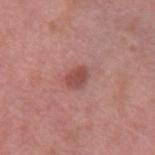Notes:
• follow-up — imaged on a skin check; not biopsied
• subject — female, aged around 40
• illumination — white-light illumination
• diameter — about 2.5 mm
• image-analysis metrics — a lesion color around L≈48 a*≈26 b*≈24 in CIELAB, roughly 10 lightness units darker than nearby skin, and a lesion-to-skin contrast of about 7.5 (normalized; higher = more distinct); border irregularity of about 2.5 on a 0–10 scale, internal color variation of about 2 on a 0–10 scale, and radial color variation of about 0.5; a classifier nevus-likeness of about 75/100 and lesion-presence confidence of about 100/100
• acquisition — ~15 mm crop, total-body skin-cancer survey
• body site — the left upper arm